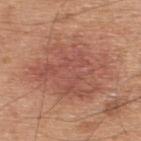<case>
  <site>upper back</site>
  <image>
    <source>total-body photography crop</source>
    <field_of_view_mm>15</field_of_view_mm>
  </image>
  <patient>
    <sex>male</sex>
    <age_approx>45</age_approx>
  </patient>
</case>